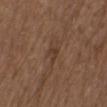* workup · catalogued during a skin exam; not biopsied
* illumination · white-light illumination
* automated lesion analysis · an area of roughly 3 mm² and an eccentricity of roughly 0.8; a lesion color around L≈37 a*≈17 b*≈27 in CIELAB and a lesion-to-skin contrast of about 6 (normalized; higher = more distinct); an automated nevus-likeness rating near 0 out of 100
* lesion size · about 2.5 mm
* location · the back
* patient · male, in their mid- to late 70s
* imaging modality · 15 mm crop, total-body photography